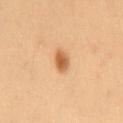  biopsy_status: not biopsied; imaged during a skin examination
  lighting: cross-polarized
  automated_metrics:
    area_mm2_approx: 4.5
    eccentricity: 0.7
    cielab_L: 49
    cielab_a: 19
    cielab_b: 34
    vs_skin_darker_L: 11.0
    vs_skin_contrast_norm: 8.0
    color_variation_0_10: 3.5
    peripheral_color_asymmetry: 1.0
    lesion_detection_confidence_0_100: 100
  site: mid back
  patient:
    sex: female
    age_approx: 40
  lesion_size:
    long_diameter_mm_approx: 2.5
  image:
    source: total-body photography crop
    field_of_view_mm: 15